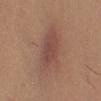No biopsy was performed on this lesion — it was imaged during a full skin examination and was not determined to be concerning.